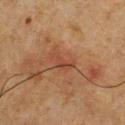follow-up = catalogued during a skin exam; not biopsied
subject = male, aged 58–62
illumination = cross-polarized illumination
image = ~15 mm crop, total-body skin-cancer survey
location = the chest